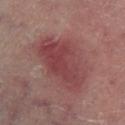Q: Is there a histopathology result?
A: total-body-photography surveillance lesion; no biopsy
Q: Automated lesion metrics?
A: an average lesion color of about L≈40 a*≈25 b*≈19 (CIELAB); a detector confidence of about 100 out of 100 that the crop contains a lesion
Q: Where on the body is the lesion?
A: the left lower leg
Q: How large is the lesion?
A: ≈7.5 mm
Q: How was this image acquired?
A: ~15 mm tile from a whole-body skin photo
Q: What lighting was used for the tile?
A: cross-polarized
Q: What are the patient's age and sex?
A: male, approximately 65 years of age